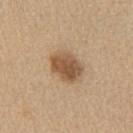Clinical impression: The lesion was photographed on a routine skin check and not biopsied; there is no pathology result. Context: Captured under white-light illumination. The lesion's longest dimension is about 4 mm. A 15 mm close-up extracted from a 3D total-body photography capture. The subject is a female aged approximately 60. The lesion is located on the left upper arm.